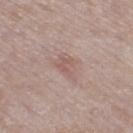Clinical impression: The lesion was photographed on a routine skin check and not biopsied; there is no pathology result. Acquisition and patient details: A female subject, aged around 60. From the right thigh. This image is a 15 mm lesion crop taken from a total-body photograph.